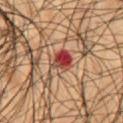Part of a total-body skin-imaging series; this lesion was reviewed on a skin check and was not flagged for biopsy. Captured under cross-polarized illumination. The subject is a male roughly 50 years of age. This image is a 15 mm lesion crop taken from a total-body photograph. The lesion is located on the mid back.